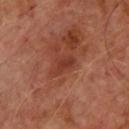Q: Was this lesion biopsied?
A: no biopsy performed (imaged during a skin exam)
Q: What are the patient's age and sex?
A: male, in their mid- to late 60s
Q: Illumination type?
A: cross-polarized illumination
Q: How large is the lesion?
A: ~3 mm (longest diameter)
Q: How was this image acquired?
A: total-body-photography crop, ~15 mm field of view
Q: What did automated image analysis measure?
A: a footprint of about 4 mm², an outline eccentricity of about 0.75 (0 = round, 1 = elongated), and a shape-asymmetry score of about 0.4 (0 = symmetric); a classifier nevus-likeness of about 0/100